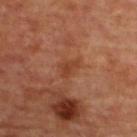Clinical impression: No biopsy was performed on this lesion — it was imaged during a full skin examination and was not determined to be concerning. Image and clinical context: A 15 mm close-up extracted from a 3D total-body photography capture. An algorithmic analysis of the crop reported an average lesion color of about L≈40 a*≈25 b*≈32 (CIELAB), about 7 CIELAB-L* units darker than the surrounding skin, and a normalized lesion–skin contrast near 6. The lesion is located on the upper back. Longest diameter approximately 2.5 mm. This is a cross-polarized tile. A female subject, in their mid- to late 40s.